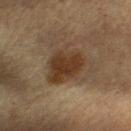Q: Was this lesion biopsied?
A: imaged on a skin check; not biopsied
Q: Lesion location?
A: the chest
Q: What did automated image analysis measure?
A: a border-irregularity index near 2.5/10 and a within-lesion color-variation index near 3.5/10
Q: Illumination type?
A: cross-polarized illumination
Q: What is the lesion's diameter?
A: ~5 mm (longest diameter)
Q: How was this image acquired?
A: total-body-photography crop, ~15 mm field of view
Q: What are the patient's age and sex?
A: male, aged 63–67